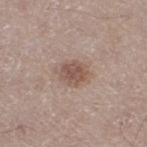workup: total-body-photography surveillance lesion; no biopsy
illumination: white-light
lesion size: about 3.5 mm
subject: female, aged 48–52
acquisition: 15 mm crop, total-body photography
TBP lesion metrics: a lesion area of about 7.5 mm², an outline eccentricity of about 0.65 (0 = round, 1 = elongated), and a symmetry-axis asymmetry near 0.2; an average lesion color of about L≈53 a*≈17 b*≈24 (CIELAB), about 10 CIELAB-L* units darker than the surrounding skin, and a lesion-to-skin contrast of about 7 (normalized; higher = more distinct); a border-irregularity rating of about 2.5/10 and a within-lesion color-variation index near 2.5/10
site: the right thigh